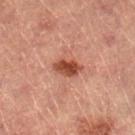| key | value |
|---|---|
| follow-up | imaged on a skin check; not biopsied |
| diameter | ~3.5 mm (longest diameter) |
| TBP lesion metrics | a footprint of about 5.5 mm², an outline eccentricity of about 0.85 (0 = round, 1 = elongated), and a shape-asymmetry score of about 0.2 (0 = symmetric); an average lesion color of about L≈40 a*≈24 b*≈28 (CIELAB) and roughly 12 lightness units darker than nearby skin; border irregularity of about 2 on a 0–10 scale, internal color variation of about 3 on a 0–10 scale, and a peripheral color-asymmetry measure near 1 |
| image source | ~15 mm tile from a whole-body skin photo |
| location | the right lower leg |
| patient | female, aged around 70 |
| lighting | cross-polarized illumination |